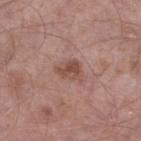The lesion was photographed on a routine skin check and not biopsied; there is no pathology result. This is a white-light tile. Automated tile analysis of the lesion measured a border-irregularity rating of about 3.5/10, a color-variation rating of about 4/10, and radial color variation of about 1.5. The analysis additionally found an automated nevus-likeness rating near 35 out of 100 and lesion-presence confidence of about 100/100. A close-up tile cropped from a whole-body skin photograph, about 15 mm across. A male patient aged 53–57. Approximately 3.5 mm at its widest. Located on the left lower leg.